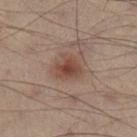notes: imaged on a skin check; not biopsied | image: total-body-photography crop, ~15 mm field of view | patient: male, approximately 55 years of age | anatomic site: the right lower leg.